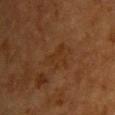Imaged during a routine full-body skin examination; the lesion was not biopsied and no histopathology is available. From the chest. The subject is a female roughly 40 years of age. Imaged with cross-polarized lighting. A close-up tile cropped from a whole-body skin photograph, about 15 mm across. An algorithmic analysis of the crop reported a footprint of about 6 mm², an outline eccentricity of about 0.7 (0 = round, 1 = elongated), and a shape-asymmetry score of about 0.55 (0 = symmetric). And it measured a border-irregularity index near 6/10, internal color variation of about 1.5 on a 0–10 scale, and a peripheral color-asymmetry measure near 0.5. And it measured a nevus-likeness score of about 0/100 and a detector confidence of about 100 out of 100 that the crop contains a lesion.